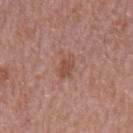<lesion>
<biopsy_status>not biopsied; imaged during a skin examination</biopsy_status>
<site>mid back</site>
<patient>
  <sex>male</sex>
  <age_approx>65</age_approx>
</patient>
<image>
  <source>total-body photography crop</source>
  <field_of_view_mm>15</field_of_view_mm>
</image>
</lesion>